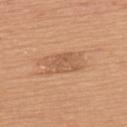Q: Was this lesion biopsied?
A: imaged on a skin check; not biopsied
Q: Who is the patient?
A: female, aged around 75
Q: Lesion location?
A: the upper back
Q: Automated lesion metrics?
A: a lesion color around L≈58 a*≈22 b*≈34 in CIELAB, a lesion–skin lightness drop of about 8, and a lesion-to-skin contrast of about 5.5 (normalized; higher = more distinct); a within-lesion color-variation index near 2.5/10 and a peripheral color-asymmetry measure near 1; a nevus-likeness score of about 15/100 and lesion-presence confidence of about 100/100
Q: How was this image acquired?
A: 15 mm crop, total-body photography
Q: Lesion size?
A: about 4.5 mm
Q: What lighting was used for the tile?
A: white-light illumination